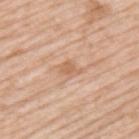{
  "biopsy_status": "not biopsied; imaged during a skin examination",
  "lesion_size": {
    "long_diameter_mm_approx": 2.5
  },
  "site": "upper back",
  "automated_metrics": {
    "nevus_likeness_0_100": 0,
    "lesion_detection_confidence_0_100": 100
  },
  "lighting": "white-light",
  "patient": {
    "sex": "male",
    "age_approx": 80
  },
  "image": {
    "source": "total-body photography crop",
    "field_of_view_mm": 15
  }
}